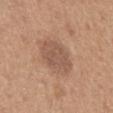Impression:
Captured during whole-body skin photography for melanoma surveillance; the lesion was not biopsied.
Clinical summary:
The total-body-photography lesion software estimated a mean CIELAB color near L≈53 a*≈19 b*≈28 and a normalized border contrast of about 6.5. The analysis additionally found a border-irregularity index near 2.5/10 and internal color variation of about 2 on a 0–10 scale. It also reported a classifier nevus-likeness of about 20/100 and a detector confidence of about 100 out of 100 that the crop contains a lesion. A 15 mm close-up tile from a total-body photography series done for melanoma screening. Located on the mid back. Measured at roughly 5 mm in maximum diameter. Imaged with white-light lighting. A male subject, in their mid-60s.